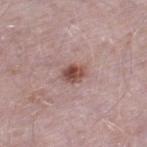Notes:
* biopsy status · catalogued during a skin exam; not biopsied
* tile lighting · white-light illumination
* patient · male, roughly 50 years of age
* imaging modality · 15 mm crop, total-body photography
* site · the left thigh